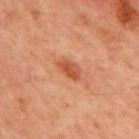<tbp_lesion>
  <biopsy_status>not biopsied; imaged during a skin examination</biopsy_status>
  <patient>
    <sex>male</sex>
    <age_approx>65</age_approx>
  </patient>
  <site>front of the torso</site>
  <lesion_size>
    <long_diameter_mm_approx>3.0</long_diameter_mm_approx>
  </lesion_size>
  <image>
    <source>total-body photography crop</source>
    <field_of_view_mm>15</field_of_view_mm>
  </image>
  <lighting>cross-polarized</lighting>
</tbp_lesion>